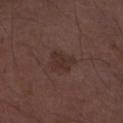notes = no biopsy performed (imaged during a skin exam)